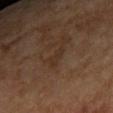{
  "biopsy_status": "not biopsied; imaged during a skin examination",
  "patient": {
    "sex": "female",
    "age_approx": 60
  },
  "image": {
    "source": "total-body photography crop",
    "field_of_view_mm": 15
  },
  "site": "left forearm"
}